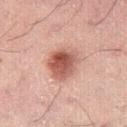Q: Was a biopsy performed?
A: imaged on a skin check; not biopsied
Q: What is the imaging modality?
A: ~15 mm crop, total-body skin-cancer survey
Q: Lesion location?
A: the right thigh
Q: Who is the patient?
A: male, aged 48 to 52
Q: Automated lesion metrics?
A: an eccentricity of roughly 0.6 and two-axis asymmetry of about 0.2; a mean CIELAB color near L≈49 a*≈23 b*≈25, about 14 CIELAB-L* units darker than the surrounding skin, and a normalized lesion–skin contrast near 9.5
Q: How large is the lesion?
A: ~4.5 mm (longest diameter)